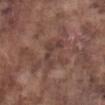The lesion was tiled from a total-body skin photograph and was not biopsied.